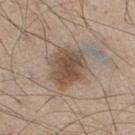– workup: catalogued during a skin exam; not biopsied
– imaging modality: total-body-photography crop, ~15 mm field of view
– anatomic site: the right thigh
– patient: male, aged 43 to 47
– lighting: white-light